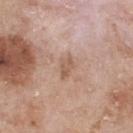| key | value |
|---|---|
| workup | catalogued during a skin exam; not biopsied |
| lighting | white-light illumination |
| subject | male, aged 53 to 57 |
| automated lesion analysis | a border-irregularity rating of about 3/10 and peripheral color asymmetry of about 0.5; a classifier nevus-likeness of about 0/100 and a lesion-detection confidence of about 100/100 |
| size | about 2.5 mm |
| imaging modality | ~15 mm crop, total-body skin-cancer survey |
| anatomic site | the upper back |